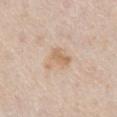Q: Was a biopsy performed?
A: no biopsy performed (imaged during a skin exam)
Q: What are the patient's age and sex?
A: male, about 75 years old
Q: What kind of image is this?
A: ~15 mm tile from a whole-body skin photo
Q: How was the tile lit?
A: white-light illumination
Q: What is the lesion's diameter?
A: ≈3.5 mm
Q: What is the anatomic site?
A: the abdomen
Q: What did automated image analysis measure?
A: a nevus-likeness score of about 0/100 and a detector confidence of about 100 out of 100 that the crop contains a lesion